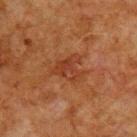Impression: Captured during whole-body skin photography for melanoma surveillance; the lesion was not biopsied. Clinical summary: A 15 mm crop from a total-body photograph taken for skin-cancer surveillance. Imaged with cross-polarized lighting. The patient is a male aged 78 to 82. About 3.5 mm across. On the back.